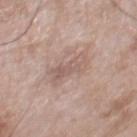Recorded during total-body skin imaging; not selected for excision or biopsy. Imaged with white-light lighting. Located on the chest. Cropped from a whole-body photographic skin survey; the tile spans about 15 mm. A male patient, aged 68 to 72. About 4.5 mm across.